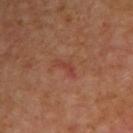Captured during whole-body skin photography for melanoma surveillance; the lesion was not biopsied. A male subject, about 55 years old. From the right upper arm. A 15 mm close-up extracted from a 3D total-body photography capture.